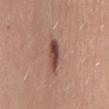<case>
<lesion_size>
  <long_diameter_mm_approx>5.0</long_diameter_mm_approx>
</lesion_size>
<patient>
  <sex>female</sex>
  <age_approx>35</age_approx>
</patient>
<image>
  <source>total-body photography crop</source>
  <field_of_view_mm>15</field_of_view_mm>
</image>
<site>abdomen</site>
</case>